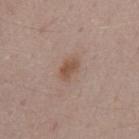Recorded during total-body skin imaging; not selected for excision or biopsy.
The total-body-photography lesion software estimated a footprint of about 4.5 mm², an eccentricity of roughly 0.8, and a symmetry-axis asymmetry near 0.2. It also reported a mean CIELAB color near L≈51 a*≈18 b*≈27, a lesion–skin lightness drop of about 9, and a lesion-to-skin contrast of about 7.5 (normalized; higher = more distinct). The analysis additionally found a border-irregularity rating of about 2/10, a color-variation rating of about 1.5/10, and a peripheral color-asymmetry measure near 0.5.
The lesion is on the mid back.
A male subject, aged around 55.
Captured under white-light illumination.
A lesion tile, about 15 mm wide, cut from a 3D total-body photograph.
About 3 mm across.